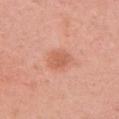Imaged during a routine full-body skin examination; the lesion was not biopsied and no histopathology is available. On the left upper arm. The subject is a female roughly 35 years of age. The recorded lesion diameter is about 3 mm. A 15 mm crop from a total-body photograph taken for skin-cancer surveillance. This is a white-light tile.